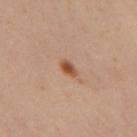The patient is a female aged 38 to 42.
The tile uses cross-polarized illumination.
A 15 mm close-up tile from a total-body photography series done for melanoma screening.
Measured at roughly 2.5 mm in maximum diameter.
From the left thigh.